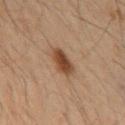Clinical impression:
Recorded during total-body skin imaging; not selected for excision or biopsy.
Context:
A male patient roughly 50 years of age. Automated tile analysis of the lesion measured a mean CIELAB color near L≈33 a*≈16 b*≈25, a lesion–skin lightness drop of about 11, and a lesion-to-skin contrast of about 10 (normalized; higher = more distinct). And it measured a nevus-likeness score of about 100/100 and a detector confidence of about 100 out of 100 that the crop contains a lesion. The lesion's longest dimension is about 3.5 mm. The lesion is on the mid back. A close-up tile cropped from a whole-body skin photograph, about 15 mm across. This is a cross-polarized tile.